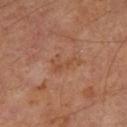Assessment:
No biopsy was performed on this lesion — it was imaged during a full skin examination and was not determined to be concerning.
Clinical summary:
The tile uses cross-polarized illumination. A male patient about 70 years old. On the right thigh. The lesion's longest dimension is about 3.5 mm. A 15 mm close-up extracted from a 3D total-body photography capture. An algorithmic analysis of the crop reported about 5 CIELAB-L* units darker than the surrounding skin and a normalized lesion–skin contrast near 5.5.